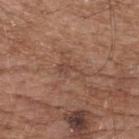{"biopsy_status": "not biopsied; imaged during a skin examination", "patient": {"sex": "male", "age_approx": 75}, "lighting": "white-light", "automated_metrics": {"cielab_L": 45, "cielab_a": 20, "cielab_b": 27, "vs_skin_darker_L": 6.0, "vs_skin_contrast_norm": 5.0, "border_irregularity_0_10": 8.0, "color_variation_0_10": 1.0, "peripheral_color_asymmetry": 0.5, "nevus_likeness_0_100": 0}, "lesion_size": {"long_diameter_mm_approx": 3.0}, "image": {"source": "total-body photography crop", "field_of_view_mm": 15}, "site": "back"}